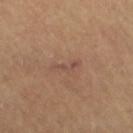Captured during whole-body skin photography for melanoma surveillance; the lesion was not biopsied. A female patient about 60 years old. The tile uses cross-polarized illumination. The lesion is located on the right thigh. A 15 mm crop from a total-body photograph taken for skin-cancer surveillance. The lesion's longest dimension is about 3.5 mm.